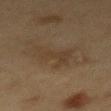* workup: total-body-photography surveillance lesion; no biopsy
* patient: male, aged approximately 60
* body site: the mid back
* lesion diameter: ~4.5 mm (longest diameter)
* image-analysis metrics: an area of roughly 9 mm² and an outline eccentricity of about 0.55 (0 = round, 1 = elongated); an average lesion color of about L≈30 a*≈11 b*≈24 (CIELAB), about 4 CIELAB-L* units darker than the surrounding skin, and a normalized border contrast of about 5; internal color variation of about 2 on a 0–10 scale and peripheral color asymmetry of about 0.5; an automated nevus-likeness rating near 0 out of 100 and a detector confidence of about 100 out of 100 that the crop contains a lesion
* imaging modality: 15 mm crop, total-body photography